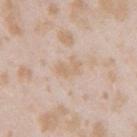Q: Is there a histopathology result?
A: total-body-photography surveillance lesion; no biopsy
Q: What is the anatomic site?
A: the right upper arm
Q: How was this image acquired?
A: total-body-photography crop, ~15 mm field of view
Q: Who is the patient?
A: female, aged around 25
Q: How large is the lesion?
A: ≈3 mm